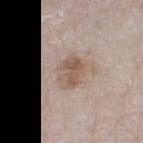Clinical impression: Captured during whole-body skin photography for melanoma surveillance; the lesion was not biopsied. Acquisition and patient details: Longest diameter approximately 4 mm. The tile uses white-light illumination. A male patient roughly 80 years of age. This image is a 15 mm lesion crop taken from a total-body photograph. The lesion is on the right lower leg.